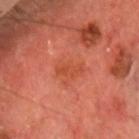Assessment:
The lesion was photographed on a routine skin check and not biopsied; there is no pathology result.
Clinical summary:
This image is a 15 mm lesion crop taken from a total-body photograph. The subject is a male aged approximately 70. The lesion is on the head or neck.